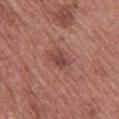Impression: This lesion was catalogued during total-body skin photography and was not selected for biopsy. Image and clinical context: Captured under white-light illumination. The lesion's longest dimension is about 3 mm. A female subject aged around 60. This image is a 15 mm lesion crop taken from a total-body photograph. The lesion is on the upper back.